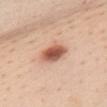Notes:
– notes · no biopsy performed (imaged during a skin exam)
– lesion diameter · ≈4 mm
– lighting · white-light illumination
– site · the chest
– subject · female, approximately 45 years of age
– imaging modality · 15 mm crop, total-body photography
– automated lesion analysis · a lesion area of about 7.5 mm², a shape eccentricity near 0.8, and a symmetry-axis asymmetry near 0.15; a border-irregularity index near 1.5/10, internal color variation of about 5 on a 0–10 scale, and radial color variation of about 1.5